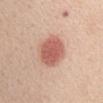Notes:
- follow-up · catalogued during a skin exam; not biopsied
- site · the chest
- lesion diameter · ≈4.5 mm
- TBP lesion metrics · a lesion area of about 14 mm² and two-axis asymmetry of about 0.15
- subject · female, roughly 50 years of age
- acquisition · 15 mm crop, total-body photography